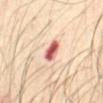Q: Was this lesion biopsied?
A: imaged on a skin check; not biopsied
Q: How was this image acquired?
A: 15 mm crop, total-body photography
Q: How large is the lesion?
A: ≈3 mm
Q: Patient demographics?
A: male, aged around 70
Q: Where on the body is the lesion?
A: the abdomen
Q: Illumination type?
A: cross-polarized